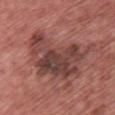Findings:
• biopsy status: imaged on a skin check; not biopsied
• size: about 8.5 mm
• body site: the chest
• patient: male, aged approximately 60
• acquisition: total-body-photography crop, ~15 mm field of view